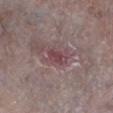notes: no biopsy performed (imaged during a skin exam)
image: total-body-photography crop, ~15 mm field of view
body site: the leg
patient: female, about 65 years old
automated lesion analysis: a lesion area of about 4 mm², an eccentricity of roughly 0.8, and a shape-asymmetry score of about 0.25 (0 = symmetric)